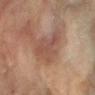– acquisition — total-body-photography crop, ~15 mm field of view
– anatomic site — the right forearm
– subject — female, about 80 years old